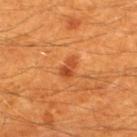Impression:
No biopsy was performed on this lesion — it was imaged during a full skin examination and was not determined to be concerning.
Acquisition and patient details:
The patient is a male aged 58 to 62. Automated tile analysis of the lesion measured a lesion area of about 3.5 mm². And it measured about 9 CIELAB-L* units darker than the surrounding skin and a lesion-to-skin contrast of about 7 (normalized; higher = more distinct). The software also gave a border-irregularity rating of about 3.5/10 and a peripheral color-asymmetry measure near 0.5. The tile uses cross-polarized illumination. A region of skin cropped from a whole-body photographic capture, roughly 15 mm wide. On the back. The lesion's longest dimension is about 3 mm.